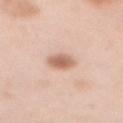Recorded during total-body skin imaging; not selected for excision or biopsy. A female subject, aged 48 to 52. About 3 mm across. Cropped from a whole-body photographic skin survey; the tile spans about 15 mm. Automated tile analysis of the lesion measured a mean CIELAB color near L≈64 a*≈20 b*≈30, a lesion–skin lightness drop of about 13, and a normalized border contrast of about 8. The analysis additionally found a color-variation rating of about 3.5/10 and radial color variation of about 1. It also reported a nevus-likeness score of about 95/100 and a detector confidence of about 100 out of 100 that the crop contains a lesion. From the mid back. Captured under white-light illumination.